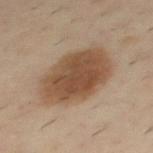workup: imaged on a skin check; not biopsied
lesion size: ≈7 mm
illumination: cross-polarized illumination
image source: 15 mm crop, total-body photography
body site: the upper back
subject: male, in their 40s